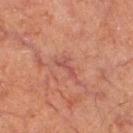| key | value |
|---|---|
| follow-up | total-body-photography surveillance lesion; no biopsy |
| lighting | cross-polarized illumination |
| site | the leg |
| subject | male, aged approximately 65 |
| lesion diameter | ≈3.5 mm |
| image | total-body-photography crop, ~15 mm field of view |